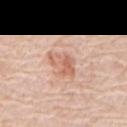Case summary:
- biopsy status · imaged on a skin check; not biopsied
- subject · male, aged around 80
- anatomic site · the mid back
- image source · ~15 mm tile from a whole-body skin photo